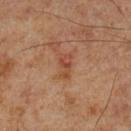Captured during whole-body skin photography for melanoma surveillance; the lesion was not biopsied.
Cropped from a total-body skin-imaging series; the visible field is about 15 mm.
An algorithmic analysis of the crop reported a footprint of about 3.5 mm², a shape eccentricity near 0.85, and two-axis asymmetry of about 0.45. The analysis additionally found peripheral color asymmetry of about 0.5. And it measured a lesion-detection confidence of about 100/100.
On the left lower leg.
A male patient aged 68 to 72.